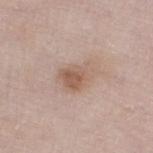Recorded during total-body skin imaging; not selected for excision or biopsy.
A male subject, about 80 years old.
The lesion's longest dimension is about 4.5 mm.
The lesion is on the leg.
A 15 mm close-up tile from a total-body photography series done for melanoma screening.
This is a white-light tile.